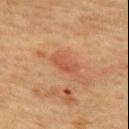The lesion was tiled from a total-body skin photograph and was not biopsied. Located on the upper back. About 3 mm across. A 15 mm close-up tile from a total-body photography series done for melanoma screening. The tile uses cross-polarized illumination. A female subject approximately 55 years of age.